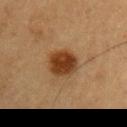Part of a total-body skin-imaging series; this lesion was reviewed on a skin check and was not flagged for biopsy. Cropped from a total-body skin-imaging series; the visible field is about 15 mm. A male patient about 55 years old. From the left upper arm.